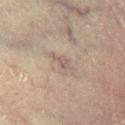biopsy status — catalogued during a skin exam; not biopsied
acquisition — total-body-photography crop, ~15 mm field of view
subject — male, roughly 70 years of age
location — the leg